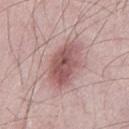• notes — catalogued during a skin exam; not biopsied
• image — ~15 mm crop, total-body skin-cancer survey
• anatomic site — the left thigh
• subject — male, about 25 years old